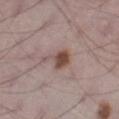follow-up: no biopsy performed (imaged during a skin exam)
lesion size: ≈3.5 mm
image-analysis metrics: about 11 CIELAB-L* units darker than the surrounding skin and a normalized border contrast of about 8.5; border irregularity of about 4.5 on a 0–10 scale, a within-lesion color-variation index near 4.5/10, and peripheral color asymmetry of about 1
anatomic site: the leg
lighting: white-light
subject: male, approximately 70 years of age
image source: ~15 mm tile from a whole-body skin photo